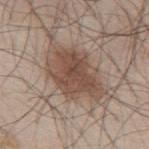Impression: The lesion was tiled from a total-body skin photograph and was not biopsied. Context: On the mid back. Cropped from a whole-body photographic skin survey; the tile spans about 15 mm. Imaged with white-light lighting. A male patient approximately 45 years of age. Automated tile analysis of the lesion measured roughly 11 lightness units darker than nearby skin and a lesion-to-skin contrast of about 8 (normalized; higher = more distinct). And it measured an automated nevus-likeness rating near 95 out of 100 and a detector confidence of about 100 out of 100 that the crop contains a lesion. Approximately 7 mm at its widest.